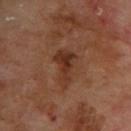Q: Is there a histopathology result?
A: imaged on a skin check; not biopsied
Q: Lesion location?
A: the back
Q: Illumination type?
A: cross-polarized
Q: How was this image acquired?
A: total-body-photography crop, ~15 mm field of view
Q: Patient demographics?
A: male, about 70 years old
Q: Lesion size?
A: about 5 mm